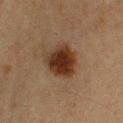Part of a total-body skin-imaging series; this lesion was reviewed on a skin check and was not flagged for biopsy.